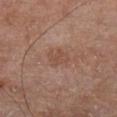Captured under cross-polarized illumination. Longest diameter approximately 2.5 mm. The lesion is on the left lower leg. Automated tile analysis of the lesion measured an area of roughly 5 mm², a shape eccentricity near 0.5, and two-axis asymmetry of about 0.25. The analysis additionally found roughly 6 lightness units darker than nearby skin and a normalized border contrast of about 5. The analysis additionally found a border-irregularity index near 2.5/10, a within-lesion color-variation index near 2/10, and radial color variation of about 0.5. And it measured a classifier nevus-likeness of about 0/100 and lesion-presence confidence of about 100/100. A lesion tile, about 15 mm wide, cut from a 3D total-body photograph. The patient is a male approximately 65 years of age.